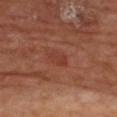Clinical impression: This lesion was catalogued during total-body skin photography and was not selected for biopsy. Acquisition and patient details: Approximately 2.5 mm at its widest. From the chest. An algorithmic analysis of the crop reported a mean CIELAB color near L≈37 a*≈26 b*≈28 and a lesion–skin lightness drop of about 5. And it measured a color-variation rating of about 2/10 and peripheral color asymmetry of about 0.5. And it measured lesion-presence confidence of about 100/100. The subject is a male in their 70s. A roughly 15 mm field-of-view crop from a total-body skin photograph. The tile uses cross-polarized illumination.